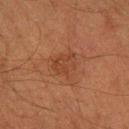Impression:
Recorded during total-body skin imaging; not selected for excision or biopsy.
Context:
The tile uses cross-polarized illumination. A 15 mm close-up tile from a total-body photography series done for melanoma screening. The lesion is located on the right forearm. A male subject, roughly 50 years of age. The lesion's longest dimension is about 3 mm.